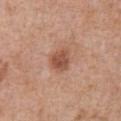{
  "biopsy_status": "not biopsied; imaged during a skin examination",
  "lesion_size": {
    "long_diameter_mm_approx": 3.0
  },
  "lighting": "white-light",
  "site": "front of the torso",
  "image": {
    "source": "total-body photography crop",
    "field_of_view_mm": 15
  },
  "patient": {
    "sex": "male",
    "age_approx": 70
  }
}